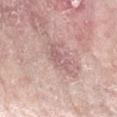  image:
    source: total-body photography crop
    field_of_view_mm: 15
  patient:
    sex: female
    age_approx: 70
  automated_metrics:
    nevus_likeness_0_100: 0
    lesion_detection_confidence_0_100: 70
  site: left forearm